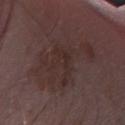Impression:
This lesion was catalogued during total-body skin photography and was not selected for biopsy.
Context:
Approximately 9.5 mm at its widest. Captured under white-light illumination. Cropped from a whole-body photographic skin survey; the tile spans about 15 mm. Located on the right forearm. A male subject aged around 75.